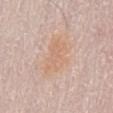biopsy_status: not biopsied; imaged during a skin examination
patient:
  sex: male
  age_approx: 75
lighting: white-light
automated_metrics:
  area_mm2_approx: 10.0
  eccentricity: 0.75
  shape_asymmetry: 0.3
  border_irregularity_0_10: 4.0
  color_variation_0_10: 2.5
  peripheral_color_asymmetry: 1.0
site: abdomen
lesion_size:
  long_diameter_mm_approx: 4.5
image:
  source: total-body photography crop
  field_of_view_mm: 15